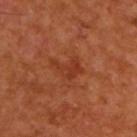Assessment:
The lesion was photographed on a routine skin check and not biopsied; there is no pathology result.
Acquisition and patient details:
The recorded lesion diameter is about 3.5 mm. A roughly 15 mm field-of-view crop from a total-body skin photograph. The tile uses cross-polarized illumination. From the upper back. Automated tile analysis of the lesion measured a lesion area of about 5.5 mm² and a symmetry-axis asymmetry near 0.55. It also reported a border-irregularity rating of about 6.5/10 and peripheral color asymmetry of about 1. The software also gave a nevus-likeness score of about 0/100. A male patient, approximately 65 years of age.